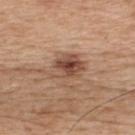{"biopsy_status": "not biopsied; imaged during a skin examination", "patient": {"sex": "male", "age_approx": 65}, "site": "upper back", "image": {"source": "total-body photography crop", "field_of_view_mm": 15}, "lesion_size": {"long_diameter_mm_approx": 4.0}, "lighting": "white-light"}